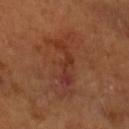Q: Was this lesion biopsied?
A: total-body-photography surveillance lesion; no biopsy
Q: What lighting was used for the tile?
A: cross-polarized illumination
Q: What is the lesion's diameter?
A: ~7.5 mm (longest diameter)
Q: What is the anatomic site?
A: the right forearm
Q: What is the imaging modality?
A: total-body-photography crop, ~15 mm field of view
Q: Who is the patient?
A: female, about 55 years old